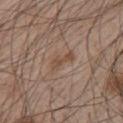biopsy status: imaged on a skin check; not biopsied
automated metrics: an area of roughly 5 mm², an eccentricity of roughly 0.9, and a symmetry-axis asymmetry near 0.45; border irregularity of about 5 on a 0–10 scale and internal color variation of about 1.5 on a 0–10 scale; an automated nevus-likeness rating near 0 out of 100 and lesion-presence confidence of about 100/100
lesion diameter: ≈3.5 mm
patient: male, aged 63–67
acquisition: total-body-photography crop, ~15 mm field of view
illumination: white-light illumination
site: the chest